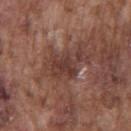Q: Was a biopsy performed?
A: total-body-photography surveillance lesion; no biopsy
Q: How was the tile lit?
A: white-light illumination
Q: Automated lesion metrics?
A: an area of roughly 9.5 mm², a shape eccentricity near 0.8, and two-axis asymmetry of about 0.45; a mean CIELAB color near L≈38 a*≈20 b*≈23, a lesion–skin lightness drop of about 8, and a normalized border contrast of about 7.5; a peripheral color-asymmetry measure near 1; a nevus-likeness score of about 0/100 and lesion-presence confidence of about 65/100
Q: How large is the lesion?
A: ≈5 mm
Q: Lesion location?
A: the chest
Q: Patient demographics?
A: male, roughly 75 years of age
Q: How was this image acquired?
A: ~15 mm crop, total-body skin-cancer survey